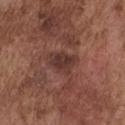Recorded during total-body skin imaging; not selected for excision or biopsy.
The lesion is located on the chest.
A roughly 15 mm field-of-view crop from a total-body skin photograph.
Automated tile analysis of the lesion measured a lesion color around L≈35 a*≈21 b*≈22 in CIELAB, a lesion–skin lightness drop of about 9, and a lesion-to-skin contrast of about 8 (normalized; higher = more distinct). The software also gave a border-irregularity rating of about 2.5/10. It also reported a lesion-detection confidence of about 100/100.
A male subject, approximately 75 years of age.